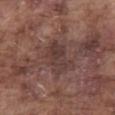Impression: Recorded during total-body skin imaging; not selected for excision or biopsy. Background: Measured at roughly 4 mm in maximum diameter. The lesion is on the abdomen. A male patient, aged 73 to 77. A lesion tile, about 15 mm wide, cut from a 3D total-body photograph. The lesion-visualizer software estimated a lesion area of about 10 mm², a shape eccentricity near 0.75, and a shape-asymmetry score of about 0.25 (0 = symmetric). The software also gave a mean CIELAB color near L≈37 a*≈17 b*≈20, a lesion–skin lightness drop of about 7, and a lesion-to-skin contrast of about 6.5 (normalized; higher = more distinct). The analysis additionally found a nevus-likeness score of about 0/100 and a lesion-detection confidence of about 95/100.